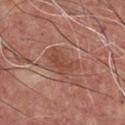Assessment:
Recorded during total-body skin imaging; not selected for excision or biopsy.
Context:
A 15 mm crop from a total-body photograph taken for skin-cancer surveillance. The lesion-visualizer software estimated a lesion area of about 6.5 mm² and an outline eccentricity of about 0.85 (0 = round, 1 = elongated). The analysis additionally found a classifier nevus-likeness of about 0/100 and a detector confidence of about 100 out of 100 that the crop contains a lesion. Located on the chest. A male patient about 65 years old. This is a white-light tile. Longest diameter approximately 4 mm.